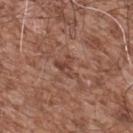Case summary:
- illumination: white-light illumination
- patient: male, aged around 55
- size: ~3 mm (longest diameter)
- TBP lesion metrics: an average lesion color of about L≈44 a*≈22 b*≈26 (CIELAB) and a lesion-to-skin contrast of about 6.5 (normalized; higher = more distinct); a border-irregularity rating of about 6/10, a within-lesion color-variation index near 3/10, and peripheral color asymmetry of about 1; an automated nevus-likeness rating near 0 out of 100 and a detector confidence of about 75 out of 100 that the crop contains a lesion
- body site: the upper back
- acquisition: ~15 mm tile from a whole-body skin photo